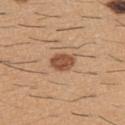The lesion was photographed on a routine skin check and not biopsied; there is no pathology result.
About 3 mm across.
On the upper back.
An algorithmic analysis of the crop reported a footprint of about 6.5 mm² and a shape-asymmetry score of about 0.2 (0 = symmetric). The analysis additionally found an average lesion color of about L≈52 a*≈22 b*≈33 (CIELAB) and roughly 13 lightness units darker than nearby skin. And it measured a border-irregularity rating of about 2/10 and peripheral color asymmetry of about 1. The software also gave an automated nevus-likeness rating near 95 out of 100.
The patient is a male in their 60s.
Imaged with white-light lighting.
A 15 mm close-up extracted from a 3D total-body photography capture.